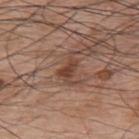Clinical impression:
No biopsy was performed on this lesion — it was imaged during a full skin examination and was not determined to be concerning.
Clinical summary:
The lesion is on the upper back. The tile uses white-light illumination. A male subject about 70 years old. The lesion's longest dimension is about 4 mm. Cropped from a whole-body photographic skin survey; the tile spans about 15 mm.